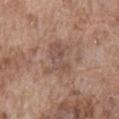Findings:
• follow-up: no biopsy performed (imaged during a skin exam)
• tile lighting: white-light
• subject: male, aged 73 to 77
• site: the abdomen
• lesion size: ~4.5 mm (longest diameter)
• image source: 15 mm crop, total-body photography